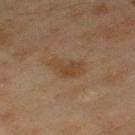Assessment:
Part of a total-body skin-imaging series; this lesion was reviewed on a skin check and was not flagged for biopsy.